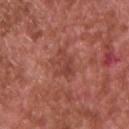- workup: total-body-photography surveillance lesion; no biopsy
- acquisition: 15 mm crop, total-body photography
- patient: male, aged 63 to 67
- body site: the chest